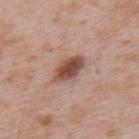Impression: This lesion was catalogued during total-body skin photography and was not selected for biopsy. Image and clinical context: From the upper back. A male patient, aged approximately 50. Cropped from a whole-body photographic skin survey; the tile spans about 15 mm.